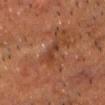biopsy status=catalogued during a skin exam; not biopsied
image source=15 mm crop, total-body photography
subject=male, aged 48–52
body site=the head or neck